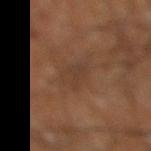Recorded during total-body skin imaging; not selected for excision or biopsy. A male patient, aged approximately 60. The lesion is on the right lower leg. Longest diameter approximately 4.5 mm. This is a cross-polarized tile. A 15 mm close-up extracted from a 3D total-body photography capture. Automated tile analysis of the lesion measured an average lesion color of about L≈34 a*≈16 b*≈25 (CIELAB), roughly 5 lightness units darker than nearby skin, and a normalized border contrast of about 5. It also reported a classifier nevus-likeness of about 0/100 and lesion-presence confidence of about 50/100.